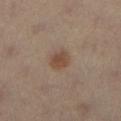biopsy status: catalogued during a skin exam; not biopsied | lesion diameter: ~2.5 mm (longest diameter) | location: the leg | patient: female, aged 38 to 42 | imaging modality: 15 mm crop, total-body photography | illumination: cross-polarized.